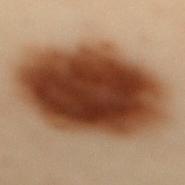image = ~15 mm crop, total-body skin-cancer survey | diameter = ~12 mm (longest diameter) | lighting = cross-polarized | subject = female, aged 58–62 | body site = the back.